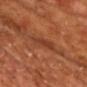Background:
Imaged with cross-polarized lighting. The subject is a male in their mid-60s. Automated tile analysis of the lesion measured a lesion area of about 5.5 mm², a shape eccentricity near 0.85, and a shape-asymmetry score of about 0.3 (0 = symmetric). The software also gave an average lesion color of about L≈39 a*≈27 b*≈33 (CIELAB) and a lesion–skin lightness drop of about 6. The analysis additionally found an automated nevus-likeness rating near 0 out of 100 and a lesion-detection confidence of about 95/100. The lesion's longest dimension is about 3.5 mm. A 15 mm crop from a total-body photograph taken for skin-cancer surveillance.